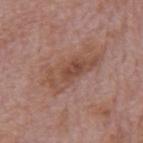Part of a total-body skin-imaging series; this lesion was reviewed on a skin check and was not flagged for biopsy. A 15 mm crop from a total-body photograph taken for skin-cancer surveillance. Captured under white-light illumination. The subject is a male aged approximately 75. The lesion is located on the mid back.